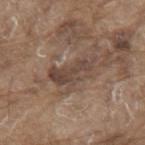Captured during whole-body skin photography for melanoma surveillance; the lesion was not biopsied. The lesion is on the back. A roughly 15 mm field-of-view crop from a total-body skin photograph. The subject is a male aged approximately 80.